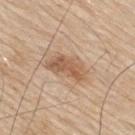Case summary:
– lesion diameter · ≈5 mm
– anatomic site · the back
– acquisition · ~15 mm tile from a whole-body skin photo
– subject · male, approximately 80 years of age
– image-analysis metrics · a lesion color around L≈58 a*≈19 b*≈33 in CIELAB, a lesion–skin lightness drop of about 11, and a normalized border contrast of about 7.5
– tile lighting · white-light illumination